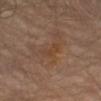Assessment:
The lesion was tiled from a total-body skin photograph and was not biopsied.
Clinical summary:
The lesion-visualizer software estimated a footprint of about 4 mm² and two-axis asymmetry of about 0.35. It also reported a border-irregularity index near 4/10. The lesion is located on the left thigh. A region of skin cropped from a whole-body photographic capture, roughly 15 mm wide. A male patient aged 63–67.